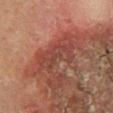biopsy status = no biopsy performed (imaged during a skin exam)
size = about 6 mm
automated lesion analysis = a footprint of about 12 mm², an eccentricity of roughly 0.9, and a symmetry-axis asymmetry near 0.4; a color-variation rating of about 3.5/10 and peripheral color asymmetry of about 1.5
tile lighting = cross-polarized
body site = the left lower leg
subject = female, approximately 50 years of age
image = ~15 mm crop, total-body skin-cancer survey